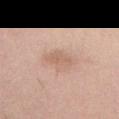notes=catalogued during a skin exam; not biopsied
subject=female, aged approximately 55
body site=the left thigh
image source=15 mm crop, total-body photography